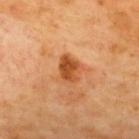Impression: Recorded during total-body skin imaging; not selected for excision or biopsy. Image and clinical context: The lesion is on the upper back. A subject roughly 70 years of age. This is a cross-polarized tile. A lesion tile, about 15 mm wide, cut from a 3D total-body photograph. The lesion's longest dimension is about 3 mm.